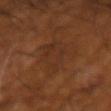workup — imaged on a skin check; not biopsied
automated lesion analysis — a footprint of about 15 mm², a shape eccentricity near 0.8, and a shape-asymmetry score of about 0.45 (0 = symmetric); a lesion color around L≈31 a*≈20 b*≈29 in CIELAB and a normalized lesion–skin contrast near 5
subject — male, in their mid- to late 60s
acquisition — 15 mm crop, total-body photography
anatomic site — the right forearm